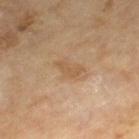Clinical impression:
Recorded during total-body skin imaging; not selected for excision or biopsy.